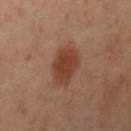{"biopsy_status": "not biopsied; imaged during a skin examination", "site": "left arm", "lighting": "cross-polarized", "image": {"source": "total-body photography crop", "field_of_view_mm": 15}, "lesion_size": {"long_diameter_mm_approx": 4.5}, "patient": {"sex": "female", "age_approx": 40}}